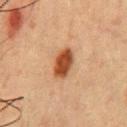The lesion was tiled from a total-body skin photograph and was not biopsied.
A region of skin cropped from a whole-body photographic capture, roughly 15 mm wide.
Approximately 4 mm at its widest.
Automated tile analysis of the lesion measured a classifier nevus-likeness of about 100/100.
A male patient roughly 50 years of age.
From the chest.
This is a cross-polarized tile.